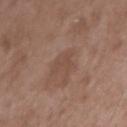Assessment: The lesion was tiled from a total-body skin photograph and was not biopsied. Context: The lesion's longest dimension is about 2.5 mm. On the right upper arm. Imaged with white-light lighting. A male patient, approximately 50 years of age. A 15 mm crop from a total-body photograph taken for skin-cancer surveillance.